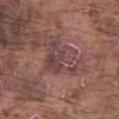Captured during whole-body skin photography for melanoma surveillance; the lesion was not biopsied. A roughly 15 mm field-of-view crop from a total-body skin photograph. A male patient aged 73–77. From the left thigh.